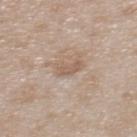The lesion was photographed on a routine skin check and not biopsied; there is no pathology result. A 15 mm close-up extracted from a 3D total-body photography capture. Automated tile analysis of the lesion measured a footprint of about 3.5 mm², a shape eccentricity near 0.85, and a shape-asymmetry score of about 0.4 (0 = symmetric). It also reported a classifier nevus-likeness of about 0/100 and a detector confidence of about 100 out of 100 that the crop contains a lesion. Measured at roughly 2.5 mm in maximum diameter. On the upper back. A female subject in their 30s.